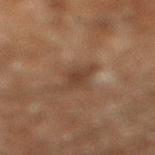notes = imaged on a skin check; not biopsied
acquisition = ~15 mm crop, total-body skin-cancer survey
body site = the right lower leg
patient = male, approximately 60 years of age
lighting = cross-polarized illumination